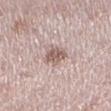The lesion was photographed on a routine skin check and not biopsied; there is no pathology result. Automated tile analysis of the lesion measured a lesion area of about 5.5 mm², an outline eccentricity of about 0.55 (0 = round, 1 = elongated), and two-axis asymmetry of about 0.25. And it measured a lesion color around L≈58 a*≈17 b*≈21 in CIELAB, roughly 12 lightness units darker than nearby skin, and a lesion-to-skin contrast of about 8 (normalized; higher = more distinct). And it measured border irregularity of about 2 on a 0–10 scale, internal color variation of about 3 on a 0–10 scale, and peripheral color asymmetry of about 1. And it measured an automated nevus-likeness rating near 20 out of 100 and a lesion-detection confidence of about 100/100. The lesion's longest dimension is about 3 mm. A female patient, approximately 45 years of age. From the left lower leg. A lesion tile, about 15 mm wide, cut from a 3D total-body photograph. Imaged with white-light lighting.